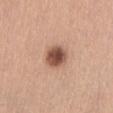workup: imaged on a skin check; not biopsied | image: ~15 mm tile from a whole-body skin photo | size: about 3 mm | automated lesion analysis: a footprint of about 7 mm², an eccentricity of roughly 0.5, and two-axis asymmetry of about 0.2; a mean CIELAB color near L≈51 a*≈22 b*≈28, about 17 CIELAB-L* units darker than the surrounding skin, and a lesion-to-skin contrast of about 11 (normalized; higher = more distinct) | subject: female, aged 53–57 | anatomic site: the abdomen | tile lighting: white-light.